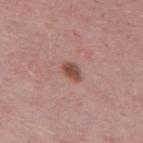Recorded during total-body skin imaging; not selected for excision or biopsy.
Captured under white-light illumination.
The lesion-visualizer software estimated an area of roughly 3.5 mm², a shape eccentricity near 0.75, and two-axis asymmetry of about 0.25.
A male patient aged approximately 50.
The lesion is located on the upper back.
This image is a 15 mm lesion crop taken from a total-body photograph.
Longest diameter approximately 2.5 mm.